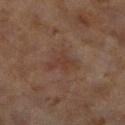Background: A female subject, roughly 60 years of age. This image is a 15 mm lesion crop taken from a total-body photograph. The lesion-visualizer software estimated a footprint of about 3.5 mm², an eccentricity of roughly 0.8, and a shape-asymmetry score of about 0.65 (0 = symmetric). Measured at roughly 3 mm in maximum diameter. On the left lower leg. Imaged with cross-polarized lighting.